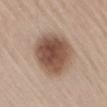Part of a total-body skin-imaging series; this lesion was reviewed on a skin check and was not flagged for biopsy.
The patient is a male aged 63–67.
This is a white-light tile.
Automated image analysis of the tile measured a footprint of about 26 mm², a shape eccentricity near 0.5, and two-axis asymmetry of about 0.1. And it measured a border-irregularity index near 1/10, a within-lesion color-variation index near 5.5/10, and peripheral color asymmetry of about 1.5. The software also gave an automated nevus-likeness rating near 95 out of 100 and lesion-presence confidence of about 100/100.
This image is a 15 mm lesion crop taken from a total-body photograph.
The lesion is on the lower back.